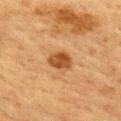workup — total-body-photography surveillance lesion; no biopsy | patient — female, aged around 55 | location — the upper back | acquisition — 15 mm crop, total-body photography.